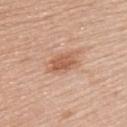The lesion was tiled from a total-body skin photograph and was not biopsied. Located on the arm. A female subject, aged 48–52. This is a white-light tile. About 3.5 mm across. This image is a 15 mm lesion crop taken from a total-body photograph.